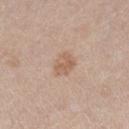Recorded during total-body skin imaging; not selected for excision or biopsy.
The lesion is on the right lower leg.
A 15 mm close-up extracted from a 3D total-body photography capture.
Longest diameter approximately 2.5 mm.
This is a white-light tile.
The total-body-photography lesion software estimated about 8 CIELAB-L* units darker than the surrounding skin and a normalized lesion–skin contrast near 6.
The patient is a female aged around 40.